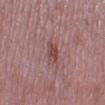Q: Was this lesion biopsied?
A: imaged on a skin check; not biopsied